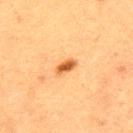Case summary:
- follow-up — catalogued during a skin exam; not biopsied
- site — the upper back
- image — 15 mm crop, total-body photography
- subject — male, about 40 years old